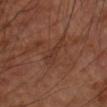biopsy_status: not biopsied; imaged during a skin examination
patient:
  sex: male
  age_approx: 70
lesion_size:
  long_diameter_mm_approx: 4.0
image:
  source: total-body photography crop
  field_of_view_mm: 15
site: left upper arm